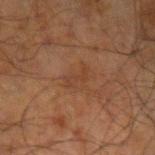| feature | finding |
|---|---|
| workup | imaged on a skin check; not biopsied |
| patient | male, about 70 years old |
| illumination | cross-polarized illumination |
| lesion diameter | ≈2.5 mm |
| location | the left upper arm |
| image source | ~15 mm tile from a whole-body skin photo |